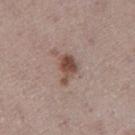Part of a total-body skin-imaging series; this lesion was reviewed on a skin check and was not flagged for biopsy. Cropped from a whole-body photographic skin survey; the tile spans about 15 mm. About 3.5 mm across. The patient is a male in their mid-70s. The lesion is on the right thigh. Imaged with white-light lighting. Automated tile analysis of the lesion measured a lesion area of about 6 mm², an eccentricity of roughly 0.8, and a symmetry-axis asymmetry near 0.4. The analysis additionally found a mean CIELAB color near L≈48 a*≈18 b*≈24 and a normalized lesion–skin contrast near 9.